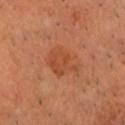{"patient": {"sex": "male", "age_approx": 65}, "site": "head or neck", "lighting": "cross-polarized", "automated_metrics": {"area_mm2_approx": 11.0, "shape_asymmetry": 0.3, "cielab_L": 45, "cielab_a": 26, "cielab_b": 35, "vs_skin_darker_L": 6.0}, "image": {"source": "total-body photography crop", "field_of_view_mm": 15}}